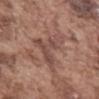Clinical impression: No biopsy was performed on this lesion — it was imaged during a full skin examination and was not determined to be concerning. Acquisition and patient details: The lesion's longest dimension is about 6.5 mm. Automated image analysis of the tile measured an area of roughly 12 mm² and two-axis asymmetry of about 0.7. The analysis additionally found border irregularity of about 9.5 on a 0–10 scale, a color-variation rating of about 2.5/10, and a peripheral color-asymmetry measure near 1. On the abdomen. Captured under white-light illumination. A 15 mm close-up extracted from a 3D total-body photography capture. The subject is a male aged approximately 75.